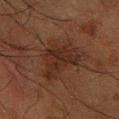{
  "biopsy_status": "not biopsied; imaged during a skin examination",
  "lesion_size": {
    "long_diameter_mm_approx": 6.0
  },
  "lighting": "cross-polarized",
  "image": {
    "source": "total-body photography crop",
    "field_of_view_mm": 15
  },
  "site": "right forearm",
  "patient": {
    "sex": "male",
    "age_approx": 60
  }
}